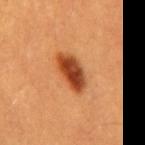Impression:
Captured during whole-body skin photography for melanoma surveillance; the lesion was not biopsied.
Context:
Cropped from a whole-body photographic skin survey; the tile spans about 15 mm. A male subject aged around 60. On the back.